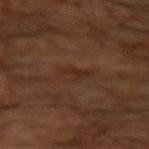Impression:
Recorded during total-body skin imaging; not selected for excision or biopsy.
Image and clinical context:
This is a cross-polarized tile. Measured at roughly 3.5 mm in maximum diameter. Located on the left arm. Cropped from a total-body skin-imaging series; the visible field is about 15 mm. A male patient aged around 60.